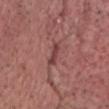Case summary:
- workup — no biopsy performed (imaged during a skin exam)